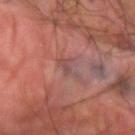Notes:
• subject — male, aged 58–62
• tile lighting — cross-polarized
• acquisition — ~15 mm tile from a whole-body skin photo
• site — the left forearm
• image-analysis metrics — a nevus-likeness score of about 0/100 and a lesion-detection confidence of about 75/100